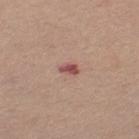follow-up = catalogued during a skin exam; not biopsied
imaging modality = ~15 mm tile from a whole-body skin photo
subject = female, approximately 45 years of age
body site = the left thigh